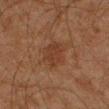The lesion was tiled from a total-body skin photograph and was not biopsied.
A male patient, aged 43 to 47.
The lesion is located on the left forearm.
A 15 mm crop from a total-body photograph taken for skin-cancer surveillance.